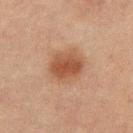notes = catalogued during a skin exam; not biopsied | lesion size = ~4.5 mm (longest diameter) | patient = male, aged approximately 65 | image = ~15 mm tile from a whole-body skin photo | site = the back | tile lighting = cross-polarized illumination.